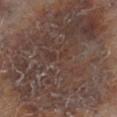Impression: Recorded during total-body skin imaging; not selected for excision or biopsy. Acquisition and patient details: The total-body-photography lesion software estimated an area of roughly 165 mm², an outline eccentricity of about 0.55 (0 = round, 1 = elongated), and a shape-asymmetry score of about 0.25 (0 = symmetric). And it measured a within-lesion color-variation index near 6/10. The patient is a female roughly 80 years of age. Imaged with cross-polarized lighting. The lesion is located on the left leg. A lesion tile, about 15 mm wide, cut from a 3D total-body photograph.